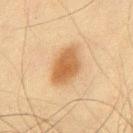Imaged during a routine full-body skin examination; the lesion was not biopsied and no histopathology is available.
The lesion's longest dimension is about 5 mm.
The tile uses cross-polarized illumination.
An algorithmic analysis of the crop reported a mean CIELAB color near L≈50 a*≈18 b*≈35, roughly 11 lightness units darker than nearby skin, and a lesion-to-skin contrast of about 8.5 (normalized; higher = more distinct). The software also gave a border-irregularity rating of about 2/10 and peripheral color asymmetry of about 1.5.
The patient is a male about 45 years old.
A roughly 15 mm field-of-view crop from a total-body skin photograph.
From the chest.